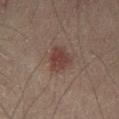No biopsy was performed on this lesion — it was imaged during a full skin examination and was not determined to be concerning. A male subject aged 38 to 42. The lesion-visualizer software estimated a lesion area of about 7.5 mm², a shape eccentricity near 0.7, and two-axis asymmetry of about 0.3. It also reported an average lesion color of about L≈35 a*≈17 b*≈20 (CIELAB), about 8 CIELAB-L* units darker than the surrounding skin, and a normalized lesion–skin contrast near 7.5. The analysis additionally found a border-irregularity rating of about 3/10, a color-variation rating of about 2/10, and a peripheral color-asymmetry measure near 0.5. It also reported a nevus-likeness score of about 100/100 and a lesion-detection confidence of about 100/100. The lesion is on the left thigh. A 15 mm close-up extracted from a 3D total-body photography capture. About 3.5 mm across.